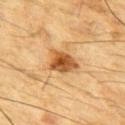Q: Was this lesion biopsied?
A: total-body-photography surveillance lesion; no biopsy
Q: Patient demographics?
A: male, aged 83 or older
Q: Where on the body is the lesion?
A: the chest
Q: What is the lesion's diameter?
A: ≈3.5 mm
Q: What kind of image is this?
A: total-body-photography crop, ~15 mm field of view
Q: Illumination type?
A: cross-polarized illumination